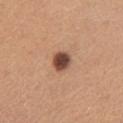Imaged during a routine full-body skin examination; the lesion was not biopsied and no histopathology is available. A 15 mm close-up extracted from a 3D total-body photography capture. Imaged with white-light lighting. From the chest. The patient is a female aged 23 to 27. Automated image analysis of the tile measured a mean CIELAB color near L≈47 a*≈22 b*≈28 and a normalized lesion–skin contrast near 12.5. The software also gave a border-irregularity rating of about 2/10, a within-lesion color-variation index near 4.5/10, and radial color variation of about 1. The analysis additionally found an automated nevus-likeness rating near 90 out of 100 and a lesion-detection confidence of about 100/100. The recorded lesion diameter is about 2.5 mm.